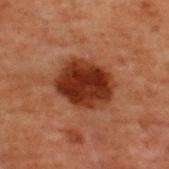Assessment: Recorded during total-body skin imaging; not selected for excision or biopsy. Background: This image is a 15 mm lesion crop taken from a total-body photograph. The lesion is located on the upper back. A male patient roughly 70 years of age. Captured under cross-polarized illumination.